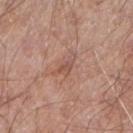| feature | finding |
|---|---|
| notes | no biopsy performed (imaged during a skin exam) |
| body site | the right forearm |
| image | 15 mm crop, total-body photography |
| subject | male, aged 63 to 67 |